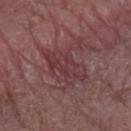Recorded during total-body skin imaging; not selected for excision or biopsy.
Located on the arm.
A region of skin cropped from a whole-body photographic capture, roughly 15 mm wide.
A male patient about 80 years old.
This is a white-light tile.